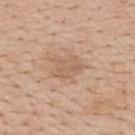Findings:
• biopsy status: no biopsy performed (imaged during a skin exam)
• imaging modality: ~15 mm tile from a whole-body skin photo
• automated metrics: an area of roughly 5.5 mm² and an outline eccentricity of about 0.7 (0 = round, 1 = elongated); an average lesion color of about L≈60 a*≈18 b*≈31 (CIELAB), about 7 CIELAB-L* units darker than the surrounding skin, and a lesion-to-skin contrast of about 5 (normalized; higher = more distinct); border irregularity of about 4 on a 0–10 scale; a nevus-likeness score of about 0/100
• size: ~3.5 mm (longest diameter)
• patient: male, aged approximately 60
• tile lighting: white-light illumination
• anatomic site: the mid back